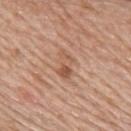Q: Was a biopsy performed?
A: imaged on a skin check; not biopsied
Q: How large is the lesion?
A: about 3.5 mm
Q: What is the imaging modality?
A: 15 mm crop, total-body photography
Q: Who is the patient?
A: female, aged 63 to 67
Q: Where on the body is the lesion?
A: the upper back
Q: How was the tile lit?
A: white-light illumination